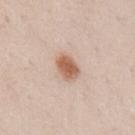Notes:
– follow-up · no biopsy performed (imaged during a skin exam)
– acquisition · ~15 mm tile from a whole-body skin photo
– patient · male, aged approximately 50
– site · the front of the torso